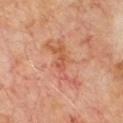This lesion was catalogued during total-body skin photography and was not selected for biopsy. Cropped from a whole-body photographic skin survey; the tile spans about 15 mm. A male subject, aged around 70. On the chest. Automated image analysis of the tile measured a footprint of about 9 mm², an outline eccentricity of about 0.95 (0 = round, 1 = elongated), and a symmetry-axis asymmetry near 0.5. The software also gave a nevus-likeness score of about 0/100 and a detector confidence of about 100 out of 100 that the crop contains a lesion. The lesion's longest dimension is about 6.5 mm.